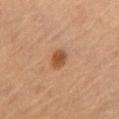Clinical impression:
Part of a total-body skin-imaging series; this lesion was reviewed on a skin check and was not flagged for biopsy.
Context:
A 15 mm close-up tile from a total-body photography series done for melanoma screening. Longest diameter approximately 3 mm. Captured under cross-polarized illumination. A female subject approximately 60 years of age. Located on the chest.